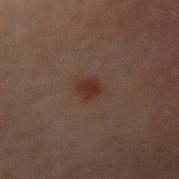Imaged during a routine full-body skin examination; the lesion was not biopsied and no histopathology is available. Automated tile analysis of the lesion measured a footprint of about 4.5 mm², an outline eccentricity of about 0.55 (0 = round, 1 = elongated), and two-axis asymmetry of about 0.2. The software also gave a border-irregularity index near 1.5/10, a within-lesion color-variation index near 2/10, and radial color variation of about 0.5. And it measured an automated nevus-likeness rating near 85 out of 100 and lesion-presence confidence of about 100/100. A 15 mm close-up extracted from a 3D total-body photography capture. The subject is a male in their 50s. Imaged with cross-polarized lighting. The lesion is located on the left arm.